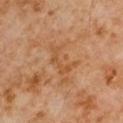{
  "biopsy_status": "not biopsied; imaged during a skin examination",
  "automated_metrics": {
    "eccentricity": 0.85,
    "shape_asymmetry": 0.7,
    "color_variation_0_10": 0.0,
    "nevus_likeness_0_100": 0
  },
  "lighting": "cross-polarized",
  "patient": {
    "sex": "male",
    "age_approx": 60
  },
  "site": "right upper arm",
  "lesion_size": {
    "long_diameter_mm_approx": 3.5
  },
  "image": {
    "source": "total-body photography crop",
    "field_of_view_mm": 15
  }
}